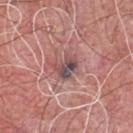Notes:
* biopsy status · catalogued during a skin exam; not biopsied
* TBP lesion metrics · about 12 CIELAB-L* units darker than the surrounding skin; border irregularity of about 3.5 on a 0–10 scale and a color-variation rating of about 9/10; an automated nevus-likeness rating near 25 out of 100 and a detector confidence of about 95 out of 100 that the crop contains a lesion
* subject · male, about 60 years old
* lesion diameter · about 3.5 mm
* lighting · white-light illumination
* image source · total-body-photography crop, ~15 mm field of view
* site · the chest